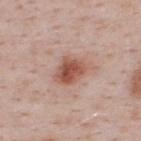Q: Was this lesion biopsied?
A: catalogued during a skin exam; not biopsied
Q: Who is the patient?
A: male, about 50 years old
Q: Automated lesion metrics?
A: an area of roughly 9 mm², an eccentricity of roughly 0.7, and a shape-asymmetry score of about 0.25 (0 = symmetric); a border-irregularity rating of about 2.5/10 and peripheral color asymmetry of about 1.5; a classifier nevus-likeness of about 95/100 and a detector confidence of about 100 out of 100 that the crop contains a lesion
Q: What is the anatomic site?
A: the upper back
Q: How large is the lesion?
A: about 4 mm
Q: Illumination type?
A: white-light
Q: What is the imaging modality?
A: ~15 mm crop, total-body skin-cancer survey